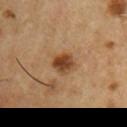Image and clinical context: The lesion is on the right upper arm. A male patient, approximately 55 years of age. A 15 mm crop from a total-body photograph taken for skin-cancer surveillance.